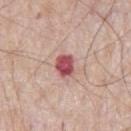Part of a total-body skin-imaging series; this lesion was reviewed on a skin check and was not flagged for biopsy.
A male patient, approximately 65 years of age.
From the front of the torso.
This image is a 15 mm lesion crop taken from a total-body photograph.
Longest diameter approximately 3 mm.